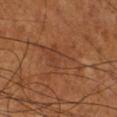Case summary:
• biopsy status · imaged on a skin check; not biopsied
• patient · male, aged 68 to 72
• imaging modality · 15 mm crop, total-body photography
• automated lesion analysis · a mean CIELAB color near L≈39 a*≈22 b*≈31, roughly 5 lightness units darker than nearby skin, and a lesion-to-skin contrast of about 4.5 (normalized; higher = more distinct); a border-irregularity index near 5/10 and internal color variation of about 3.5 on a 0–10 scale; an automated nevus-likeness rating near 0 out of 100 and a detector confidence of about 85 out of 100 that the crop contains a lesion
• lesion size · about 7 mm
• anatomic site · the right lower leg